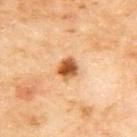The lesion was photographed on a routine skin check and not biopsied; there is no pathology result. About 3 mm across. Cropped from a total-body skin-imaging series; the visible field is about 15 mm. Located on the upper back. The lesion-visualizer software estimated a footprint of about 5 mm² and a shape-asymmetry score of about 0.2 (0 = symmetric). The software also gave a mean CIELAB color near L≈57 a*≈26 b*≈43, a lesion–skin lightness drop of about 19, and a normalized border contrast of about 11.5. The analysis additionally found a border-irregularity rating of about 2/10 and internal color variation of about 5 on a 0–10 scale. It also reported a classifier nevus-likeness of about 100/100. A female patient aged approximately 60. This is a cross-polarized tile.